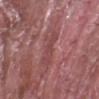Q: Was a biopsy performed?
A: imaged on a skin check; not biopsied
Q: What kind of image is this?
A: 15 mm crop, total-body photography
Q: What are the patient's age and sex?
A: male, aged 38 to 42
Q: How large is the lesion?
A: ~4 mm (longest diameter)
Q: Where on the body is the lesion?
A: the left forearm
Q: What lighting was used for the tile?
A: white-light